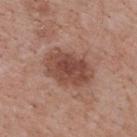Case summary:
* notes — total-body-photography surveillance lesion; no biopsy
* anatomic site — the back
* size — ~6 mm (longest diameter)
* imaging modality — ~15 mm tile from a whole-body skin photo
* tile lighting — white-light
* subject — male, aged 68 to 72